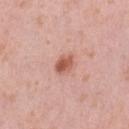A female subject in their 40s. A close-up tile cropped from a whole-body skin photograph, about 15 mm across. The tile uses white-light illumination. Longest diameter approximately 2.5 mm. The lesion is on the left thigh.